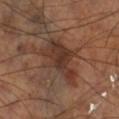Clinical summary: A 15 mm close-up extracted from a 3D total-body photography capture. Imaged with cross-polarized lighting. The subject is a male aged 68–72. Automated tile analysis of the lesion measured a footprint of about 19 mm², an outline eccentricity of about 0.8 (0 = round, 1 = elongated), and two-axis asymmetry of about 0.45. It also reported a classifier nevus-likeness of about 0/100. The lesion is located on the right lower leg. About 7.5 mm across.